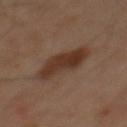Background:
This is a cross-polarized tile. The total-body-photography lesion software estimated an automated nevus-likeness rating near 95 out of 100. The subject is a male approximately 45 years of age. A lesion tile, about 15 mm wide, cut from a 3D total-body photograph. The lesion is on the chest.